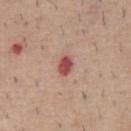The lesion was tiled from a total-body skin photograph and was not biopsied. The lesion's longest dimension is about 2.5 mm. This is a white-light tile. From the chest. The total-body-photography lesion software estimated an area of roughly 4 mm², a shape eccentricity near 0.75, and two-axis asymmetry of about 0.2. The analysis additionally found about 14 CIELAB-L* units darker than the surrounding skin and a normalized border contrast of about 10. The software also gave a classifier nevus-likeness of about 0/100 and a lesion-detection confidence of about 100/100. A male patient approximately 60 years of age. Cropped from a total-body skin-imaging series; the visible field is about 15 mm.